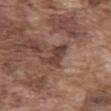notes = total-body-photography surveillance lesion; no biopsy | image source = 15 mm crop, total-body photography | site = the abdomen | subject = male, in their mid- to late 70s.